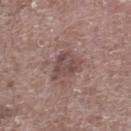notes — total-body-photography surveillance lesion; no biopsy | location — the right lower leg | subject — male, approximately 70 years of age | lesion diameter — about 3.5 mm | image — ~15 mm tile from a whole-body skin photo.